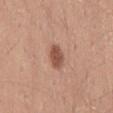notes: no biopsy performed (imaged during a skin exam) | subject: male, roughly 25 years of age | lesion size: ≈3 mm | site: the back | TBP lesion metrics: a mean CIELAB color near L≈52 a*≈23 b*≈29, a lesion–skin lightness drop of about 12, and a lesion-to-skin contrast of about 8.5 (normalized; higher = more distinct); a border-irregularity index near 1.5/10, a color-variation rating of about 2/10, and radial color variation of about 1; a classifier nevus-likeness of about 95/100 | image: ~15 mm tile from a whole-body skin photo.